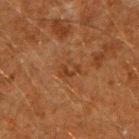Findings:
– notes: total-body-photography surveillance lesion; no biopsy
– body site: the right upper arm
– subject: male, roughly 60 years of age
– lesion size: ≈3 mm
– tile lighting: cross-polarized illumination
– acquisition: ~15 mm crop, total-body skin-cancer survey
– automated metrics: an average lesion color of about L≈31 a*≈18 b*≈29 (CIELAB), roughly 5 lightness units darker than nearby skin, and a normalized lesion–skin contrast near 5; border irregularity of about 3.5 on a 0–10 scale and a color-variation rating of about 4/10; lesion-presence confidence of about 100/100